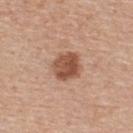Located on the back.
A 15 mm close-up tile from a total-body photography series done for melanoma screening.
A male patient, aged 53–57.